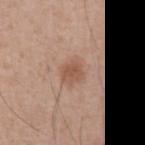Q: What lighting was used for the tile?
A: white-light illumination
Q: Lesion size?
A: ≈3 mm
Q: What kind of image is this?
A: ~15 mm tile from a whole-body skin photo
Q: What is the anatomic site?
A: the chest
Q: Patient demographics?
A: male, aged 53 to 57